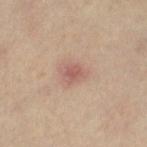Q: Was this lesion biopsied?
A: imaged on a skin check; not biopsied
Q: Where on the body is the lesion?
A: the left lower leg
Q: How was this image acquired?
A: total-body-photography crop, ~15 mm field of view
Q: Patient demographics?
A: female, aged around 35
Q: How large is the lesion?
A: ~2.5 mm (longest diameter)